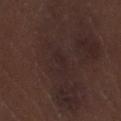workup = imaged on a skin check; not biopsied
location = the leg
subject = male, approximately 70 years of age
image = 15 mm crop, total-body photography
automated lesion analysis = an area of roughly 3 mm², an eccentricity of roughly 0.9, and two-axis asymmetry of about 0.4; a lesion color around L≈23 a*≈14 b*≈14 in CIELAB, about 3 CIELAB-L* units darker than the surrounding skin, and a normalized lesion–skin contrast near 4.5; a border-irregularity index near 4/10, a within-lesion color-variation index near 1/10, and a peripheral color-asymmetry measure near 0.5; a classifier nevus-likeness of about 0/100
diameter = about 3 mm
tile lighting = white-light